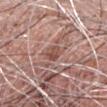Assessment:
This lesion was catalogued during total-body skin photography and was not selected for biopsy.
Clinical summary:
From the head or neck. This is a white-light tile. A lesion tile, about 15 mm wide, cut from a 3D total-body photograph. A male patient, aged 73 to 77. Longest diameter approximately 3 mm.